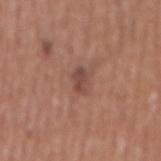Part of a total-body skin-imaging series; this lesion was reviewed on a skin check and was not flagged for biopsy. A roughly 15 mm field-of-view crop from a total-body skin photograph. The lesion is on the abdomen. A male subject, in their mid- to late 40s. The tile uses white-light illumination.